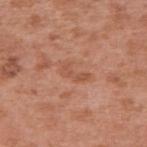Assessment: The lesion was tiled from a total-body skin photograph and was not biopsied. Image and clinical context: Cropped from a whole-body photographic skin survey; the tile spans about 15 mm. An algorithmic analysis of the crop reported a lesion area of about 4 mm². The software also gave a mean CIELAB color near L≈53 a*≈25 b*≈33, about 7 CIELAB-L* units darker than the surrounding skin, and a normalized lesion–skin contrast near 5. It also reported border irregularity of about 4.5 on a 0–10 scale, a color-variation rating of about 0.5/10, and radial color variation of about 0. The software also gave a classifier nevus-likeness of about 0/100 and a lesion-detection confidence of about 100/100. A female patient aged 38 to 42. On the arm. This is a white-light tile. The recorded lesion diameter is about 3.5 mm.